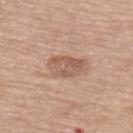patient: male, roughly 75 years of age
site: the upper back
diameter: ~4 mm (longest diameter)
tile lighting: white-light illumination
acquisition: total-body-photography crop, ~15 mm field of view